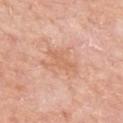From the right upper arm.
Automated image analysis of the tile measured a lesion color around L≈64 a*≈23 b*≈34 in CIELAB, about 7 CIELAB-L* units darker than the surrounding skin, and a lesion-to-skin contrast of about 5.5 (normalized; higher = more distinct).
Measured at roughly 4 mm in maximum diameter.
The subject is a female about 65 years old.
A roughly 15 mm field-of-view crop from a total-body skin photograph.
This is a white-light tile.